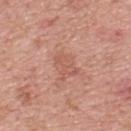biopsy status = no biopsy performed (imaged during a skin exam)
site = the upper back
subject = female, aged 38–42
tile lighting = white-light
diameter = about 3 mm
image source = 15 mm crop, total-body photography